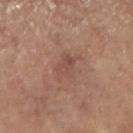<case>
<biopsy_status>not biopsied; imaged during a skin examination</biopsy_status>
<image>
  <source>total-body photography crop</source>
  <field_of_view_mm>15</field_of_view_mm>
</image>
<lesion_size>
  <long_diameter_mm_approx>3.5</long_diameter_mm_approx>
</lesion_size>
<site>right upper arm</site>
<patient>
  <sex>female</sex>
  <age_approx>65</age_approx>
</patient>
</case>